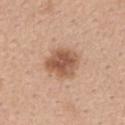  site: upper back
  patient:
    sex: female
    age_approx: 40
  image:
    source: total-body photography crop
    field_of_view_mm: 15
  lighting: white-light